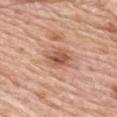Captured during whole-body skin photography for melanoma surveillance; the lesion was not biopsied. A close-up tile cropped from a whole-body skin photograph, about 15 mm across. The patient is a female in their mid-60s. This is a white-light tile. The lesion-visualizer software estimated an area of roughly 7 mm² and two-axis asymmetry of about 0.25. And it measured a mean CIELAB color near L≈56 a*≈24 b*≈31. It also reported a lesion-detection confidence of about 100/100. The lesion is on the upper back. Approximately 3.5 mm at its widest.